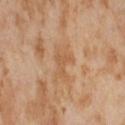| field | value |
|---|---|
| notes | imaged on a skin check; not biopsied |
| lesion diameter | ~4.5 mm (longest diameter) |
| image source | 15 mm crop, total-body photography |
| illumination | cross-polarized |
| body site | the right thigh |
| subject | female, about 55 years old |